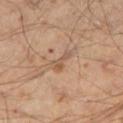Assessment:
Captured during whole-body skin photography for melanoma surveillance; the lesion was not biopsied.
Background:
Captured under cross-polarized illumination. The subject is a male aged approximately 45. Longest diameter approximately 3 mm. On the leg. This image is a 15 mm lesion crop taken from a total-body photograph.